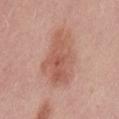Located on the back. A female patient, roughly 30 years of age. A region of skin cropped from a whole-body photographic capture, roughly 15 mm wide. The recorded lesion diameter is about 7 mm. Imaged with white-light lighting.